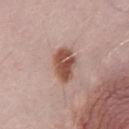acquisition: 15 mm crop, total-body photography
patient: male, aged 28–32